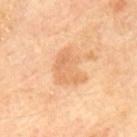Q: Was a biopsy performed?
A: imaged on a skin check; not biopsied
Q: Patient demographics?
A: male, aged approximately 70
Q: What is the anatomic site?
A: the mid back
Q: What kind of image is this?
A: ~15 mm tile from a whole-body skin photo